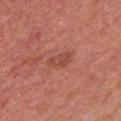Part of a total-body skin-imaging series; this lesion was reviewed on a skin check and was not flagged for biopsy.